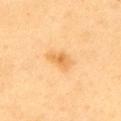Assessment:
Imaged during a routine full-body skin examination; the lesion was not biopsied and no histopathology is available.
Clinical summary:
The tile uses cross-polarized illumination. This image is a 15 mm lesion crop taken from a total-body photograph. A male patient, about 60 years old. Measured at roughly 3.5 mm in maximum diameter. Automated image analysis of the tile measured a footprint of about 6 mm², an eccentricity of roughly 0.8, and a symmetry-axis asymmetry near 0.25. The software also gave an average lesion color of about L≈71 a*≈22 b*≈48 (CIELAB), roughly 9 lightness units darker than nearby skin, and a normalized border contrast of about 6. It also reported border irregularity of about 2.5 on a 0–10 scale and radial color variation of about 0.5. It also reported a lesion-detection confidence of about 100/100. The lesion is on the left upper arm.